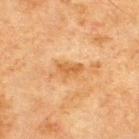Captured during whole-body skin photography for melanoma surveillance; the lesion was not biopsied.
A male patient about 70 years old.
The tile uses cross-polarized illumination.
A region of skin cropped from a whole-body photographic capture, roughly 15 mm wide.
Located on the upper back.